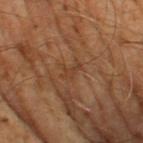Impression: The lesion was tiled from a total-body skin photograph and was not biopsied. Background: Automated image analysis of the tile measured a border-irregularity rating of about 4.5/10. Cropped from a whole-body photographic skin survey; the tile spans about 15 mm. The patient is a male aged 63–67. Imaged with cross-polarized lighting. Longest diameter approximately 2.5 mm.